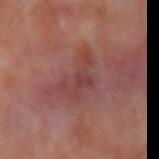Q: What are the patient's age and sex?
A: female, aged 53–57
Q: What kind of image is this?
A: 15 mm crop, total-body photography
Q: Lesion size?
A: ≈4.5 mm
Q: Where on the body is the lesion?
A: the right upper arm
Q: What did automated image analysis measure?
A: a border-irregularity rating of about 7.5/10 and a peripheral color-asymmetry measure near 0.5; a classifier nevus-likeness of about 0/100 and a lesion-detection confidence of about 95/100